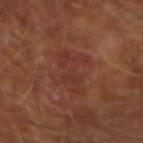Captured during whole-body skin photography for melanoma surveillance; the lesion was not biopsied.
A male patient, approximately 65 years of age.
The lesion is located on the arm.
This image is a 15 mm lesion crop taken from a total-body photograph.
This is a cross-polarized tile.